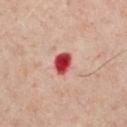Assessment: No biopsy was performed on this lesion — it was imaged during a full skin examination and was not determined to be concerning. Clinical summary: This image is a 15 mm lesion crop taken from a total-body photograph. The subject is a male roughly 65 years of age. On the chest.